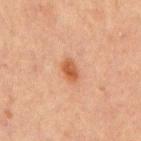A male patient, aged approximately 65. The lesion is on the chest. Captured under cross-polarized illumination. A 15 mm close-up extracted from a 3D total-body photography capture.